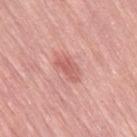No biopsy was performed on this lesion — it was imaged during a full skin examination and was not determined to be concerning. The patient is a female aged approximately 70. A close-up tile cropped from a whole-body skin photograph, about 15 mm across. About 3 mm across. This is a white-light tile. The lesion is located on the right thigh.